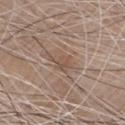Recorded during total-body skin imaging; not selected for excision or biopsy. The tile uses white-light illumination. The recorded lesion diameter is about 3 mm. A 15 mm close-up tile from a total-body photography series done for melanoma screening. An algorithmic analysis of the crop reported about 7 CIELAB-L* units darker than the surrounding skin and a lesion-to-skin contrast of about 5 (normalized; higher = more distinct). And it measured an automated nevus-likeness rating near 0 out of 100 and a lesion-detection confidence of about 50/100. Located on the front of the torso. The subject is a male about 80 years old.